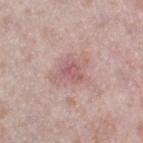biopsy status=catalogued during a skin exam; not biopsied
patient=female, in their 40s
tile lighting=white-light illumination
size=about 4 mm
image source=~15 mm tile from a whole-body skin photo
body site=the left thigh